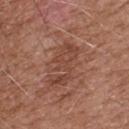  biopsy_status: not biopsied; imaged during a skin examination
  lesion_size:
    long_diameter_mm_approx: 5.5
  site: upper back
  image:
    source: total-body photography crop
    field_of_view_mm: 15
  patient:
    sex: male
    age_approx: 75